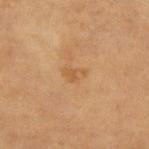Image and clinical context: The lesion is on the leg. Approximately 2.5 mm at its widest. Cropped from a total-body skin-imaging series; the visible field is about 15 mm. Imaged with cross-polarized lighting. A female subject, in their 70s.